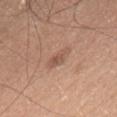{"biopsy_status": "not biopsied; imaged during a skin examination", "site": "chest", "lighting": "white-light", "patient": {"sex": "male", "age_approx": 55}, "image": {"source": "total-body photography crop", "field_of_view_mm": 15}, "lesion_size": {"long_diameter_mm_approx": 3.5}}